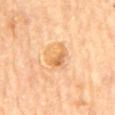Impression: The lesion was photographed on a routine skin check and not biopsied; there is no pathology result. Context: Located on the mid back. A region of skin cropped from a whole-body photographic capture, roughly 15 mm wide. The subject is a male roughly 85 years of age or older.